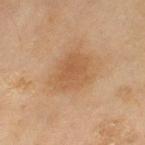Q: Is there a histopathology result?
A: total-body-photography surveillance lesion; no biopsy
Q: How large is the lesion?
A: ≈5.5 mm
Q: Patient demographics?
A: female, aged approximately 60
Q: How was the tile lit?
A: cross-polarized illumination
Q: Lesion location?
A: the left thigh
Q: How was this image acquired?
A: ~15 mm tile from a whole-body skin photo
Q: What did automated image analysis measure?
A: a border-irregularity index near 3/10, a color-variation rating of about 2/10, and radial color variation of about 0.5; an automated nevus-likeness rating near 5 out of 100 and a lesion-detection confidence of about 100/100